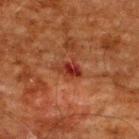site=the upper back; acquisition=~15 mm crop, total-body skin-cancer survey; tile lighting=cross-polarized illumination; patient=male, aged 58 to 62.